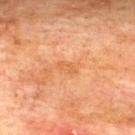{
  "biopsy_status": "not biopsied; imaged during a skin examination",
  "lighting": "cross-polarized",
  "site": "upper back",
  "patient": {
    "sex": "male",
    "age_approx": 75
  },
  "image": {
    "source": "total-body photography crop",
    "field_of_view_mm": 15
  },
  "automated_metrics": {
    "nevus_likeness_0_100": 0
  }
}